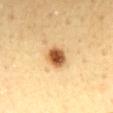Q: Is there a histopathology result?
A: catalogued during a skin exam; not biopsied